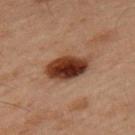The lesion was photographed on a routine skin check and not biopsied; there is no pathology result. Cropped from a total-body skin-imaging series; the visible field is about 15 mm. A male patient, aged 58 to 62. Located on the left upper arm. The lesion's longest dimension is about 5 mm.